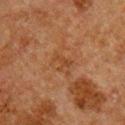Assessment: No biopsy was performed on this lesion — it was imaged during a full skin examination and was not determined to be concerning. Image and clinical context: A 15 mm close-up tile from a total-body photography series done for melanoma screening. Captured under cross-polarized illumination. A male patient, aged 58–62. From the chest. Measured at roughly 2.5 mm in maximum diameter.